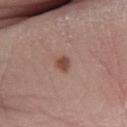Findings:
* location — the right lower leg
* TBP lesion metrics — a mean CIELAB color near L≈47 a*≈21 b*≈26, about 11 CIELAB-L* units darker than the surrounding skin, and a normalized lesion–skin contrast near 9
* subject — male, approximately 60 years of age
* imaging modality — total-body-photography crop, ~15 mm field of view